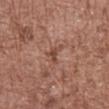  biopsy_status: not biopsied; imaged during a skin examination
  image:
    source: total-body photography crop
    field_of_view_mm: 15
  lighting: white-light
  patient:
    sex: male
    age_approx: 75
  site: chest
  lesion_size:
    long_diameter_mm_approx: 3.0